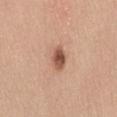Q: Was a biopsy performed?
A: catalogued during a skin exam; not biopsied
Q: Patient demographics?
A: female, aged around 40
Q: Lesion size?
A: ~3 mm (longest diameter)
Q: Automated lesion metrics?
A: a mean CIELAB color near L≈53 a*≈22 b*≈30, a lesion–skin lightness drop of about 15, and a normalized border contrast of about 10; border irregularity of about 2 on a 0–10 scale, a color-variation rating of about 4/10, and peripheral color asymmetry of about 1; a detector confidence of about 100 out of 100 that the crop contains a lesion
Q: What is the anatomic site?
A: the right thigh
Q: How was this image acquired?
A: total-body-photography crop, ~15 mm field of view
Q: What lighting was used for the tile?
A: white-light illumination